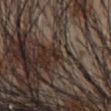Part of a total-body skin-imaging series; this lesion was reviewed on a skin check and was not flagged for biopsy.
A 15 mm crop from a total-body photograph taken for skin-cancer surveillance.
A male subject aged 53–57.
Imaged with white-light lighting.
About 1.5 mm across.
Located on the abdomen.